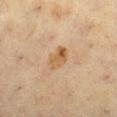Notes:
– biopsy status: no biopsy performed (imaged during a skin exam)
– body site: the leg
– patient: female, about 55 years old
– illumination: cross-polarized
– image source: total-body-photography crop, ~15 mm field of view